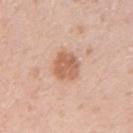| feature | finding |
|---|---|
| follow-up | imaged on a skin check; not biopsied |
| lesion diameter | ~3.5 mm (longest diameter) |
| acquisition | total-body-photography crop, ~15 mm field of view |
| automated metrics | border irregularity of about 1.5 on a 0–10 scale, a within-lesion color-variation index near 2.5/10, and a peripheral color-asymmetry measure near 1; a lesion-detection confidence of about 100/100 |
| subject | female, aged around 30 |
| location | the left upper arm |
| illumination | white-light |